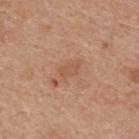workup = imaged on a skin check; not biopsied
acquisition = total-body-photography crop, ~15 mm field of view
subject = male, aged approximately 65
diameter = ~2.5 mm (longest diameter)
body site = the upper back
image-analysis metrics = a lesion color around L≈55 a*≈22 b*≈31 in CIELAB
lighting = white-light